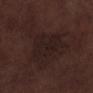Clinical impression:
This lesion was catalogued during total-body skin photography and was not selected for biopsy.
Clinical summary:
A male patient about 70 years old. The lesion is on the left lower leg. A close-up tile cropped from a whole-body skin photograph, about 15 mm across.